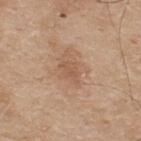Imaged during a routine full-body skin examination; the lesion was not biopsied and no histopathology is available.
A close-up tile cropped from a whole-body skin photograph, about 15 mm across.
A male patient aged 73 to 77.
The lesion is located on the upper back.
Automated tile analysis of the lesion measured a lesion color around L≈55 a*≈20 b*≈31 in CIELAB and roughly 7 lightness units darker than nearby skin. The analysis additionally found border irregularity of about 4.5 on a 0–10 scale, a within-lesion color-variation index near 1/10, and peripheral color asymmetry of about 0.5.
The tile uses white-light illumination.
Longest diameter approximately 3 mm.